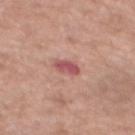Clinical impression:
Captured during whole-body skin photography for melanoma surveillance; the lesion was not biopsied.
Context:
The patient is a female in their mid-70s. Measured at roughly 2.5 mm in maximum diameter. The tile uses white-light illumination. A 15 mm close-up tile from a total-body photography series done for melanoma screening. Automated image analysis of the tile measured an eccentricity of roughly 0.8 and a symmetry-axis asymmetry near 0.25. The analysis additionally found a normalized lesion–skin contrast near 8.5. The analysis additionally found border irregularity of about 2.5 on a 0–10 scale, a within-lesion color-variation index near 1.5/10, and radial color variation of about 0.5. The software also gave an automated nevus-likeness rating near 5 out of 100 and a detector confidence of about 100 out of 100 that the crop contains a lesion. Located on the mid back.